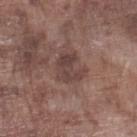{"biopsy_status": "not biopsied; imaged during a skin examination", "patient": {"sex": "male", "age_approx": 75}, "image": {"source": "total-body photography crop", "field_of_view_mm": 15}, "site": "left lower leg"}